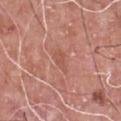Case summary:
– follow-up · total-body-photography surveillance lesion; no biopsy
– lesion size · ~2.5 mm (longest diameter)
– body site · the chest
– tile lighting · white-light
– image source · ~15 mm tile from a whole-body skin photo
– patient · male, about 60 years old